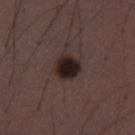The lesion was photographed on a routine skin check and not biopsied; there is no pathology result. A male patient aged 28–32. A roughly 15 mm field-of-view crop from a total-body skin photograph. The lesion is on the left thigh.